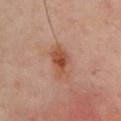Image and clinical context:
A male subject, in their mid- to late 60s. Measured at roughly 4 mm in maximum diameter. The tile uses cross-polarized illumination. On the chest. A 15 mm crop from a total-body photograph taken for skin-cancer surveillance.